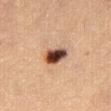subject: female, roughly 30 years of age | image source: ~15 mm tile from a whole-body skin photo | tile lighting: cross-polarized illumination | body site: the abdomen | size: ~3.5 mm (longest diameter) | automated lesion analysis: an area of roughly 7 mm², a shape eccentricity near 0.8, and a shape-asymmetry score of about 0.15 (0 = symmetric); an average lesion color of about L≈42 a*≈19 b*≈27 (CIELAB), roughly 21 lightness units darker than nearby skin, and a normalized lesion–skin contrast near 15; a border-irregularity index near 1.5/10, a within-lesion color-variation index near 8.5/10, and a peripheral color-asymmetry measure near 2.5.